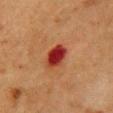The lesion was tiled from a total-body skin photograph and was not biopsied. The recorded lesion diameter is about 3.5 mm. A 15 mm close-up tile from a total-body photography series done for melanoma screening. A female patient aged around 50. On the chest. This is a cross-polarized tile.